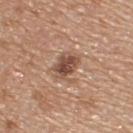biopsy status: no biopsy performed (imaged during a skin exam)